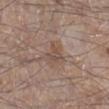Impression:
Recorded during total-body skin imaging; not selected for excision or biopsy.
Context:
The total-body-photography lesion software estimated a border-irregularity rating of about 4.5/10. And it measured an automated nevus-likeness rating near 0 out of 100 and a lesion-detection confidence of about 100/100. A 15 mm close-up extracted from a 3D total-body photography capture. About 3 mm across. The patient is a male in their mid-50s. The lesion is on the leg. Captured under white-light illumination.